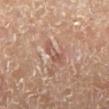Clinical summary: Longest diameter approximately 3.5 mm. A 15 mm crop from a total-body photograph taken for skin-cancer surveillance. The lesion is located on the right lower leg. A male subject, in their mid- to late 60s. Automated image analysis of the tile measured a border-irregularity rating of about 4/10, a color-variation rating of about 3/10, and peripheral color asymmetry of about 0.5.